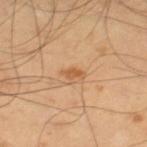{"biopsy_status": "not biopsied; imaged during a skin examination", "automated_metrics": {"area_mm2_approx": 2.5, "eccentricity": 0.85, "cielab_L": 58, "cielab_a": 23, "cielab_b": 40, "vs_skin_contrast_norm": 7.0, "border_irregularity_0_10": 2.5, "color_variation_0_10": 1.5, "peripheral_color_asymmetry": 0.5}, "site": "right thigh", "image": {"source": "total-body photography crop", "field_of_view_mm": 15}, "patient": {"sex": "male", "age_approx": 55}, "lesion_size": {"long_diameter_mm_approx": 2.5}}